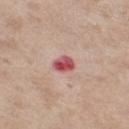{
  "biopsy_status": "not biopsied; imaged during a skin examination",
  "site": "right thigh",
  "image": {
    "source": "total-body photography crop",
    "field_of_view_mm": 15
  },
  "automated_metrics": {
    "area_mm2_approx": 4.0,
    "eccentricity": 0.6,
    "shape_asymmetry": 0.25,
    "lesion_detection_confidence_0_100": 100
  },
  "lighting": "white-light",
  "lesion_size": {
    "long_diameter_mm_approx": 2.5
  },
  "patient": {
    "sex": "female",
    "age_approx": 55
  }
}